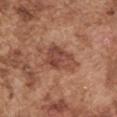notes: total-body-photography surveillance lesion; no biopsy
image source: ~15 mm crop, total-body skin-cancer survey
subject: male, aged around 75
lighting: white-light
body site: the left upper arm